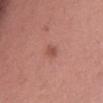Q: Was this lesion biopsied?
A: no biopsy performed (imaged during a skin exam)
Q: Lesion location?
A: the left thigh
Q: How was this image acquired?
A: 15 mm crop, total-body photography
Q: Patient demographics?
A: female, roughly 50 years of age
Q: How large is the lesion?
A: ~2.5 mm (longest diameter)
Q: How was the tile lit?
A: white-light illumination